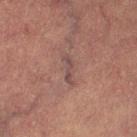The lesion was tiled from a total-body skin photograph and was not biopsied. This image is a 15 mm lesion crop taken from a total-body photograph. Measured at roughly 3 mm in maximum diameter. The patient is a female roughly 70 years of age. From the left lower leg. An algorithmic analysis of the crop reported a lesion area of about 2.5 mm², an eccentricity of roughly 0.95, and two-axis asymmetry of about 0.5. The software also gave an average lesion color of about L≈38 a*≈16 b*≈17 (CIELAB) and a normalized border contrast of about 7. It also reported a classifier nevus-likeness of about 0/100. Captured under cross-polarized illumination.